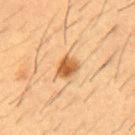Notes:
– follow-up: imaged on a skin check; not biopsied
– automated lesion analysis: a lesion area of about 5 mm², an outline eccentricity of about 0.7 (0 = round, 1 = elongated), and a symmetry-axis asymmetry near 0.25; a border-irregularity index near 2/10 and a within-lesion color-variation index near 4/10; a classifier nevus-likeness of about 95/100 and a lesion-detection confidence of about 100/100
– site: the front of the torso
– illumination: cross-polarized illumination
– patient: male, aged 53–57
– imaging modality: ~15 mm crop, total-body skin-cancer survey
– size: ~3 mm (longest diameter)